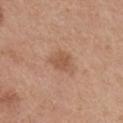Q: Is there a histopathology result?
A: imaged on a skin check; not biopsied
Q: What did automated image analysis measure?
A: a footprint of about 6 mm² and a shape-asymmetry score of about 0.3 (0 = symmetric); a lesion–skin lightness drop of about 8; a nevus-likeness score of about 25/100 and a lesion-detection confidence of about 100/100
Q: How large is the lesion?
A: ≈3.5 mm
Q: What kind of image is this?
A: total-body-photography crop, ~15 mm field of view
Q: What are the patient's age and sex?
A: female, aged 38 to 42
Q: Illumination type?
A: white-light illumination
Q: What is the anatomic site?
A: the mid back